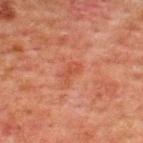Part of a total-body skin-imaging series; this lesion was reviewed on a skin check and was not flagged for biopsy. A male patient, about 60 years old. The lesion is located on the upper back. Automated image analysis of the tile measured border irregularity of about 4 on a 0–10 scale, a color-variation rating of about 1/10, and a peripheral color-asymmetry measure near 0. A 15 mm close-up extracted from a 3D total-body photography capture. Approximately 2.5 mm at its widest.